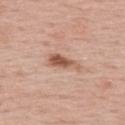Impression: The lesion was tiled from a total-body skin photograph and was not biopsied. Context: The lesion's longest dimension is about 4 mm. This is a white-light tile. Automated tile analysis of the lesion measured an area of roughly 5.5 mm², an eccentricity of roughly 0.95, and a symmetry-axis asymmetry near 0.4. The analysis additionally found roughly 13 lightness units darker than nearby skin. And it measured internal color variation of about 3 on a 0–10 scale and peripheral color asymmetry of about 1. The software also gave lesion-presence confidence of about 100/100. A male patient, about 55 years old. A close-up tile cropped from a whole-body skin photograph, about 15 mm across. The lesion is located on the upper back.